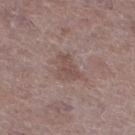Notes:
* notes — no biopsy performed (imaged during a skin exam)
* imaging modality — ~15 mm tile from a whole-body skin photo
* lighting — white-light
* diameter — ~3.5 mm (longest diameter)
* patient — male, in their mid-60s
* location — the leg